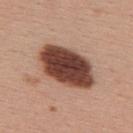{"biopsy_status": "not biopsied; imaged during a skin examination", "lighting": "white-light", "image": {"source": "total-body photography crop", "field_of_view_mm": 15}, "patient": {"sex": "female", "age_approx": 55}, "site": "upper back", "automated_metrics": {"area_mm2_approx": 23.0, "eccentricity": 0.8, "shape_asymmetry": 0.15, "cielab_L": 42, "cielab_a": 22, "cielab_b": 26, "vs_skin_darker_L": 23.0, "vs_skin_contrast_norm": 15.5, "nevus_likeness_0_100": 95}, "lesion_size": {"long_diameter_mm_approx": 7.0}}